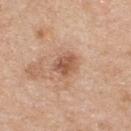Recorded during total-body skin imaging; not selected for excision or biopsy.
From the upper back.
The subject is a male roughly 60 years of age.
Cropped from a whole-body photographic skin survey; the tile spans about 15 mm.